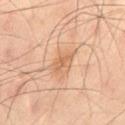The lesion was photographed on a routine skin check and not biopsied; there is no pathology result.
A 15 mm close-up extracted from a 3D total-body photography capture.
About 3.5 mm across.
The lesion is on the mid back.
The tile uses cross-polarized illumination.
The patient is a male about 40 years old.